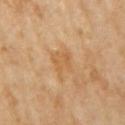The lesion was photographed on a routine skin check and not biopsied; there is no pathology result. The lesion is on the right upper arm. Cropped from a whole-body photographic skin survey; the tile spans about 15 mm. A female patient, aged 68–72.